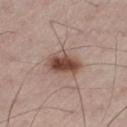Imaged during a routine full-body skin examination; the lesion was not biopsied and no histopathology is available. On the left thigh. Cropped from a total-body skin-imaging series; the visible field is about 15 mm. The lesion's longest dimension is about 4 mm. A male patient roughly 75 years of age.